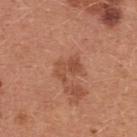A lesion tile, about 15 mm wide, cut from a 3D total-body photograph. This is a white-light tile. Longest diameter approximately 3 mm. The lesion is on the chest. Automated tile analysis of the lesion measured a classifier nevus-likeness of about 0/100 and a detector confidence of about 100 out of 100 that the crop contains a lesion. A female patient about 35 years old.